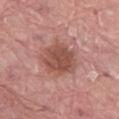Assessment:
Imaged during a routine full-body skin examination; the lesion was not biopsied and no histopathology is available.
Context:
A roughly 15 mm field-of-view crop from a total-body skin photograph. A male subject about 40 years old. The lesion is on the abdomen. The total-body-photography lesion software estimated a lesion area of about 16 mm², an eccentricity of roughly 0.5, and a symmetry-axis asymmetry near 0.2. The software also gave a classifier nevus-likeness of about 5/100 and lesion-presence confidence of about 100/100. Measured at roughly 5 mm in maximum diameter. Captured under white-light illumination.